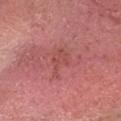  biopsy_status: not biopsied; imaged during a skin examination
  site: head or neck
  patient:
    sex: male
    age_approx: 65
  image:
    source: total-body photography crop
    field_of_view_mm: 15